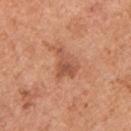A lesion tile, about 15 mm wide, cut from a 3D total-body photograph. The patient is a male about 55 years old. The total-body-photography lesion software estimated a lesion area of about 6.5 mm² and two-axis asymmetry of about 0.55. And it measured about 10 CIELAB-L* units darker than the surrounding skin. The analysis additionally found border irregularity of about 6 on a 0–10 scale, internal color variation of about 3 on a 0–10 scale, and a peripheral color-asymmetry measure near 1. Captured under white-light illumination. Measured at roughly 3.5 mm in maximum diameter. The lesion is on the chest.